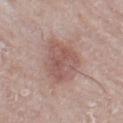follow-up: imaged on a skin check; not biopsied | lesion diameter: ~5 mm (longest diameter) | image: 15 mm crop, total-body photography | patient: female, roughly 55 years of age | location: the left thigh.